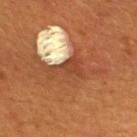Imaged during a routine full-body skin examination; the lesion was not biopsied and no histopathology is available. Measured at roughly 4 mm in maximum diameter. The patient is a female aged approximately 30. From the back. This image is a 15 mm lesion crop taken from a total-body photograph. The tile uses cross-polarized illumination.